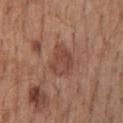Captured during whole-body skin photography for melanoma surveillance; the lesion was not biopsied. Imaged with white-light lighting. A male patient, in their 60s. On the mid back. The recorded lesion diameter is about 4 mm. The lesion-visualizer software estimated a shape eccentricity near 0.7. And it measured internal color variation of about 3 on a 0–10 scale and radial color variation of about 1. And it measured a classifier nevus-likeness of about 15/100. A close-up tile cropped from a whole-body skin photograph, about 15 mm across.